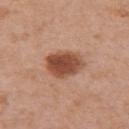Part of a total-body skin-imaging series; this lesion was reviewed on a skin check and was not flagged for biopsy.
A female patient, aged 48 to 52.
Located on the left upper arm.
This image is a 15 mm lesion crop taken from a total-body photograph.
The tile uses white-light illumination.
Automated image analysis of the tile measured a border-irregularity index near 2/10, internal color variation of about 4.5 on a 0–10 scale, and radial color variation of about 1.5. The software also gave an automated nevus-likeness rating near 100 out of 100 and a lesion-detection confidence of about 100/100.
Longest diameter approximately 4.5 mm.